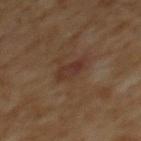This lesion was catalogued during total-body skin photography and was not selected for biopsy. The recorded lesion diameter is about 3 mm. The patient is a male aged 58–62. Captured under cross-polarized illumination. On the back. A region of skin cropped from a whole-body photographic capture, roughly 15 mm wide.